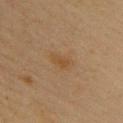workup=catalogued during a skin exam; not biopsied | image=15 mm crop, total-body photography | patient=female, aged approximately 45 | anatomic site=the back | lesion diameter=≈3 mm | tile lighting=cross-polarized illumination.